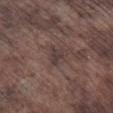Q: Was a biopsy performed?
A: no biopsy performed (imaged during a skin exam)
Q: What is the anatomic site?
A: the right lower leg
Q: How large is the lesion?
A: ≈2.5 mm
Q: What is the imaging modality?
A: ~15 mm crop, total-body skin-cancer survey
Q: What lighting was used for the tile?
A: white-light illumination
Q: What are the patient's age and sex?
A: male, about 75 years old